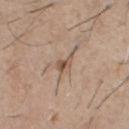{
  "biopsy_status": "not biopsied; imaged during a skin examination"
}